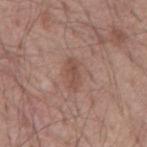biopsy status: catalogued during a skin exam; not biopsied
size: ~3.5 mm (longest diameter)
subject: male, aged approximately 55
location: the back
image: ~15 mm tile from a whole-body skin photo
illumination: white-light illumination